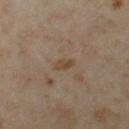{
  "biopsy_status": "not biopsied; imaged during a skin examination",
  "lighting": "cross-polarized",
  "automated_metrics": {
    "area_mm2_approx": 2.5,
    "eccentricity": 0.9,
    "shape_asymmetry": 0.3,
    "cielab_L": 42,
    "cielab_a": 14,
    "cielab_b": 28,
    "vs_skin_darker_L": 7.0,
    "vs_skin_contrast_norm": 7.0,
    "border_irregularity_0_10": 3.0,
    "color_variation_0_10": 0.0,
    "nevus_likeness_0_100": 0,
    "lesion_detection_confidence_0_100": 95
  },
  "patient": {
    "sex": "female",
    "age_approx": 35
  },
  "site": "left thigh",
  "image": {
    "source": "total-body photography crop",
    "field_of_view_mm": 15
  },
  "lesion_size": {
    "long_diameter_mm_approx": 2.5
  }
}